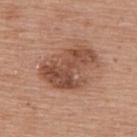biopsy status = total-body-photography surveillance lesion; no biopsy | subject = female, aged 58 to 62 | tile lighting = white-light illumination | lesion size = ~6.5 mm (longest diameter) | image = ~15 mm crop, total-body skin-cancer survey | TBP lesion metrics = an average lesion color of about L≈50 a*≈22 b*≈29 (CIELAB) and a lesion–skin lightness drop of about 12; a color-variation rating of about 6/10 and radial color variation of about 2; a classifier nevus-likeness of about 25/100 and a lesion-detection confidence of about 100/100 | location = the upper back.